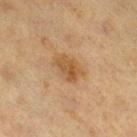Findings:
• workup · catalogued during a skin exam; not biopsied
• subject · female, approximately 40 years of age
• anatomic site · the right thigh
• image · ~15 mm crop, total-body skin-cancer survey